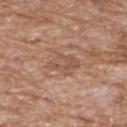A 15 mm close-up extracted from a 3D total-body photography capture. The recorded lesion diameter is about 3.5 mm. Imaged with white-light lighting. A male patient, roughly 60 years of age. The lesion is on the front of the torso.